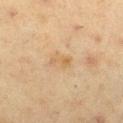Clinical impression:
Recorded during total-body skin imaging; not selected for excision or biopsy.
Context:
Cropped from a total-body skin-imaging series; the visible field is about 15 mm. Imaged with cross-polarized lighting. The recorded lesion diameter is about 2.5 mm. A female subject, about 50 years old. The lesion is on the leg.